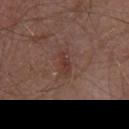| key | value |
|---|---|
| notes | catalogued during a skin exam; not biopsied |
| lesion diameter | ~3 mm (longest diameter) |
| image | ~15 mm crop, total-body skin-cancer survey |
| illumination | white-light |
| subject | male, roughly 55 years of age |
| site | the front of the torso |